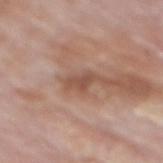The lesion was photographed on a routine skin check and not biopsied; there is no pathology result. This image is a 15 mm lesion crop taken from a total-body photograph. The lesion is on the back. Captured under white-light illumination. A male subject, aged approximately 75.